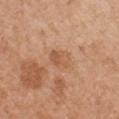Impression: The lesion was tiled from a total-body skin photograph and was not biopsied. Clinical summary: Located on the chest. The lesion's longest dimension is about 2.5 mm. This image is a 15 mm lesion crop taken from a total-body photograph. Imaged with white-light lighting. The subject is a female about 40 years old. The total-body-photography lesion software estimated border irregularity of about 1.5 on a 0–10 scale and radial color variation of about 1.5.